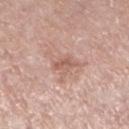patient — female, in their mid-50s; anatomic site — the left lower leg; imaging modality — 15 mm crop, total-body photography.